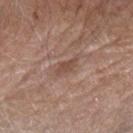Recorded during total-body skin imaging; not selected for excision or biopsy. The tile uses white-light illumination. A region of skin cropped from a whole-body photographic capture, roughly 15 mm wide. The lesion is on the left forearm. Automated tile analysis of the lesion measured a lesion area of about 3.5 mm², an eccentricity of roughly 0.9, and two-axis asymmetry of about 0.3. It also reported a lesion color around L≈48 a*≈19 b*≈26 in CIELAB, a lesion–skin lightness drop of about 8, and a normalized border contrast of about 6. The analysis additionally found an automated nevus-likeness rating near 0 out of 100 and a lesion-detection confidence of about 90/100. Measured at roughly 3 mm in maximum diameter. A male patient roughly 85 years of age or older.